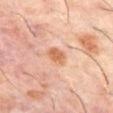• TBP lesion metrics: an average lesion color of about L≈61 a*≈22 b*≈34 (CIELAB), about 8 CIELAB-L* units darker than the surrounding skin, and a normalized lesion–skin contrast near 7
• image: total-body-photography crop, ~15 mm field of view
• location: the abdomen
• lighting: cross-polarized illumination
• patient: male, aged approximately 60